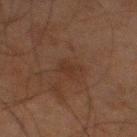Q: Was a biopsy performed?
A: total-body-photography surveillance lesion; no biopsy
Q: Who is the patient?
A: male, aged approximately 80
Q: What is the anatomic site?
A: the left thigh
Q: What kind of image is this?
A: total-body-photography crop, ~15 mm field of view